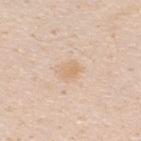Findings:
– image — ~15 mm tile from a whole-body skin photo
– location — the back
– size — about 2.5 mm
– patient — male, aged approximately 35
– automated lesion analysis — an average lesion color of about L≈71 a*≈16 b*≈34 (CIELAB), roughly 6 lightness units darker than nearby skin, and a normalized border contrast of about 5.5; internal color variation of about 2 on a 0–10 scale and peripheral color asymmetry of about 0.5; an automated nevus-likeness rating near 0 out of 100 and a detector confidence of about 100 out of 100 that the crop contains a lesion